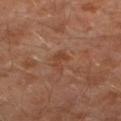Part of a total-body skin-imaging series; this lesion was reviewed on a skin check and was not flagged for biopsy. The tile uses cross-polarized illumination. A region of skin cropped from a whole-body photographic capture, roughly 15 mm wide. The subject is a male aged 28–32. An algorithmic analysis of the crop reported an area of roughly 3 mm² and an eccentricity of roughly 0.85. It also reported an average lesion color of about L≈41 a*≈22 b*≈31 (CIELAB) and a normalized border contrast of about 6. And it measured a border-irregularity rating of about 5.5/10, a within-lesion color-variation index near 0.5/10, and a peripheral color-asymmetry measure near 0. From the left lower leg. Measured at roughly 2.5 mm in maximum diameter.